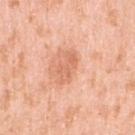{
  "biopsy_status": "not biopsied; imaged during a skin examination",
  "patient": {
    "sex": "female",
    "age_approx": 40
  },
  "image": {
    "source": "total-body photography crop",
    "field_of_view_mm": 15
  },
  "lesion_size": {
    "long_diameter_mm_approx": 3.5
  },
  "site": "right upper arm",
  "automated_metrics": {
    "shape_asymmetry": 0.35,
    "border_irregularity_0_10": 4.5,
    "color_variation_0_10": 2.0
  },
  "lighting": "white-light"
}